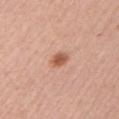workup = catalogued during a skin exam; not biopsied
lighting = white-light illumination
image source = total-body-photography crop, ~15 mm field of view
body site = the left upper arm
patient = female, aged around 50
lesion diameter = ~2.5 mm (longest diameter)